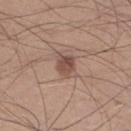<tbp_lesion>
  <biopsy_status>not biopsied; imaged during a skin examination</biopsy_status>
  <lighting>white-light</lighting>
  <patient>
    <sex>male</sex>
    <age_approx>30</age_approx>
  </patient>
  <image>
    <source>total-body photography crop</source>
    <field_of_view_mm>15</field_of_view_mm>
  </image>
  <lesion_size>
    <long_diameter_mm_approx>3.0</long_diameter_mm_approx>
  </lesion_size>
  <automated_metrics>
    <vs_skin_darker_L>11.0</vs_skin_darker_L>
    <vs_skin_contrast_norm>8.0</vs_skin_contrast_norm>
    <color_variation_0_10>3.5</color_variation_0_10>
    <peripheral_color_asymmetry>1.5</peripheral_color_asymmetry>
    <nevus_likeness_0_100>80</nevus_likeness_0_100>
    <lesion_detection_confidence_0_100>100</lesion_detection_confidence_0_100>
  </automated_metrics>
  <site>right lower leg</site>
</tbp_lesion>